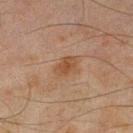Q: Was a biopsy performed?
A: catalogued during a skin exam; not biopsied